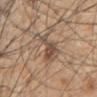Assessment: This lesion was catalogued during total-body skin photography and was not selected for biopsy. Acquisition and patient details: A lesion tile, about 15 mm wide, cut from a 3D total-body photograph. This is a white-light tile. Located on the chest. The total-body-photography lesion software estimated a lesion area of about 8.5 mm² and a symmetry-axis asymmetry near 0.6. It also reported a mean CIELAB color near L≈50 a*≈16 b*≈27 and a normalized lesion–skin contrast near 7.5. The analysis additionally found a classifier nevus-likeness of about 15/100 and lesion-presence confidence of about 95/100. A male patient, aged 48 to 52.